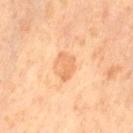Recorded during total-body skin imaging; not selected for excision or biopsy. The tile uses cross-polarized illumination. The subject is a female approximately 65 years of age. Cropped from a total-body skin-imaging series; the visible field is about 15 mm. From the left thigh.